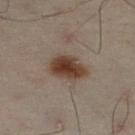Assessment:
Imaged during a routine full-body skin examination; the lesion was not biopsied and no histopathology is available.
Acquisition and patient details:
The lesion's longest dimension is about 4.5 mm. The lesion is located on the left leg. A close-up tile cropped from a whole-body skin photograph, about 15 mm across. The patient is a male approximately 50 years of age. Captured under cross-polarized illumination.